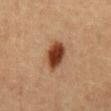notes=imaged on a skin check; not biopsied | patient=male, aged 48–52 | acquisition=total-body-photography crop, ~15 mm field of view | lesion diameter=about 4 mm | image-analysis metrics=a footprint of about 8.5 mm² and two-axis asymmetry of about 0.15 | illumination=cross-polarized illumination | body site=the abdomen.